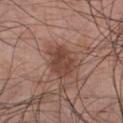Imaged during a routine full-body skin examination; the lesion was not biopsied and no histopathology is available.
A male patient, aged around 30.
On the front of the torso.
Measured at roughly 4.5 mm in maximum diameter.
The total-body-photography lesion software estimated a lesion area of about 11 mm², an outline eccentricity of about 0.7 (0 = round, 1 = elongated), and a shape-asymmetry score of about 0.2 (0 = symmetric). The software also gave a border-irregularity index near 2.5/10 and peripheral color asymmetry of about 1.
A 15 mm close-up tile from a total-body photography series done for melanoma screening.